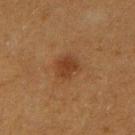This lesion was catalogued during total-body skin photography and was not selected for biopsy. A close-up tile cropped from a whole-body skin photograph, about 15 mm across. A female subject, in their 40s. Located on the right upper arm.